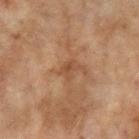Q: Is there a histopathology result?
A: imaged on a skin check; not biopsied
Q: Who is the patient?
A: female, aged 58–62
Q: Lesion location?
A: the left forearm
Q: What kind of image is this?
A: ~15 mm crop, total-body skin-cancer survey